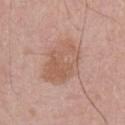follow-up: no biopsy performed (imaged during a skin exam)
patient: male, about 65 years old
lesion diameter: about 5.5 mm
image: ~15 mm tile from a whole-body skin photo
illumination: white-light illumination
anatomic site: the chest